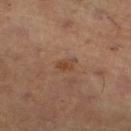Impression: Captured during whole-body skin photography for melanoma surveillance; the lesion was not biopsied. Acquisition and patient details: Automated tile analysis of the lesion measured a mean CIELAB color near L≈43 a*≈21 b*≈31 and a normalized lesion–skin contrast near 6.5. It also reported a classifier nevus-likeness of about 40/100 and lesion-presence confidence of about 100/100. A 15 mm close-up extracted from a 3D total-body photography capture. The lesion is on the left lower leg. A female patient, about 40 years old. The tile uses cross-polarized illumination.